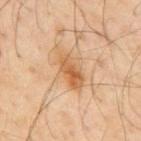Impression: No biopsy was performed on this lesion — it was imaged during a full skin examination and was not determined to be concerning. Image and clinical context: On the mid back. A roughly 15 mm field-of-view crop from a total-body skin photograph. A male subject roughly 55 years of age. Captured under cross-polarized illumination. The total-body-photography lesion software estimated a border-irregularity index near 3.5/10, a color-variation rating of about 7/10, and peripheral color asymmetry of about 2. It also reported lesion-presence confidence of about 100/100. Longest diameter approximately 5 mm.